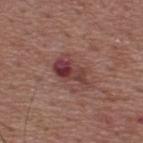The lesion was photographed on a routine skin check and not biopsied; there is no pathology result.
A male subject roughly 55 years of age.
The lesion's longest dimension is about 4.5 mm.
A lesion tile, about 15 mm wide, cut from a 3D total-body photograph.
Located on the upper back.